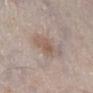Q: Is there a histopathology result?
A: no biopsy performed (imaged during a skin exam)
Q: Illumination type?
A: white-light illumination
Q: What kind of image is this?
A: 15 mm crop, total-body photography
Q: What are the patient's age and sex?
A: male, aged approximately 60
Q: Where on the body is the lesion?
A: the right lower leg
Q: Lesion size?
A: about 4 mm
Q: Automated lesion metrics?
A: an area of roughly 8 mm² and an eccentricity of roughly 0.75